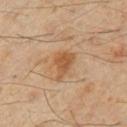This lesion was catalogued during total-body skin photography and was not selected for biopsy. The subject is a male in their 60s. The lesion-visualizer software estimated a shape eccentricity near 0.8 and two-axis asymmetry of about 0.35. The analysis additionally found a mean CIELAB color near L≈50 a*≈19 b*≈35 and a lesion–skin lightness drop of about 9. It also reported a border-irregularity index near 4/10, a color-variation rating of about 2/10, and radial color variation of about 0.5. The software also gave lesion-presence confidence of about 100/100. Imaged with cross-polarized lighting. About 4 mm across. The lesion is on the abdomen. A lesion tile, about 15 mm wide, cut from a 3D total-body photograph.